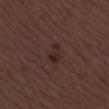Impression:
This lesion was catalogued during total-body skin photography and was not selected for biopsy.
Background:
Captured under white-light illumination. The lesion is on the left thigh. A female patient, aged 48 to 52. Longest diameter approximately 2.5 mm. A 15 mm close-up tile from a total-body photography series done for melanoma screening.